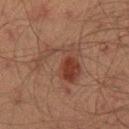Q: Is there a histopathology result?
A: catalogued during a skin exam; not biopsied